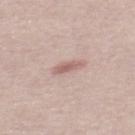biopsy status: imaged on a skin check; not biopsied
subject: male, approximately 30 years of age
illumination: white-light illumination
lesion diameter: ~3 mm (longest diameter)
image: ~15 mm tile from a whole-body skin photo
image-analysis metrics: a footprint of about 4 mm², an eccentricity of roughly 0.9, and a shape-asymmetry score of about 0.3 (0 = symmetric)